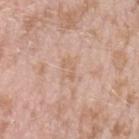Imaged during a routine full-body skin examination; the lesion was not biopsied and no histopathology is available.
The lesion is located on the left upper arm.
The subject is a female aged 23–27.
A close-up tile cropped from a whole-body skin photograph, about 15 mm across.
Longest diameter approximately 2.5 mm.